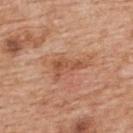This lesion was catalogued during total-body skin photography and was not selected for biopsy.
A male subject, approximately 60 years of age.
A lesion tile, about 15 mm wide, cut from a 3D total-body photograph.
The tile uses white-light illumination.
The lesion is on the upper back.
The recorded lesion diameter is about 4.5 mm.